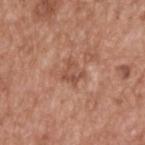Q: Was a biopsy performed?
A: no biopsy performed (imaged during a skin exam)
Q: What is the imaging modality?
A: 15 mm crop, total-body photography
Q: Illumination type?
A: white-light
Q: Who is the patient?
A: male, approximately 65 years of age
Q: How large is the lesion?
A: about 3 mm
Q: Where on the body is the lesion?
A: the back
Q: What did automated image analysis measure?
A: a footprint of about 5.5 mm² and a symmetry-axis asymmetry near 0.35; a lesion–skin lightness drop of about 8 and a normalized lesion–skin contrast near 6; a border-irregularity rating of about 4/10, a within-lesion color-variation index near 3/10, and radial color variation of about 1